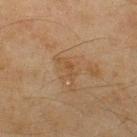follow-up: no biopsy performed (imaged during a skin exam) | tile lighting: cross-polarized | site: the upper back | patient: male, in their mid-40s | image: ~15 mm tile from a whole-body skin photo | automated metrics: a lesion–skin lightness drop of about 5 and a lesion-to-skin contrast of about 5.5 (normalized; higher = more distinct).